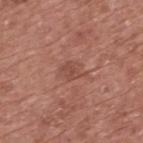The recorded lesion diameter is about 3 mm.
From the upper back.
A 15 mm crop from a total-body photograph taken for skin-cancer surveillance.
This is a white-light tile.
A male patient in their mid-70s.